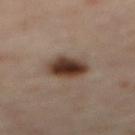Clinical impression: The lesion was tiled from a total-body skin photograph and was not biopsied. Acquisition and patient details: Approximately 4 mm at its widest. The lesion is located on the mid back. A lesion tile, about 15 mm wide, cut from a 3D total-body photograph. The patient is a female approximately 60 years of age. The tile uses cross-polarized illumination.